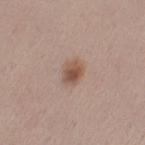Case summary:
* biopsy status — total-body-photography surveillance lesion; no biopsy
* tile lighting — white-light illumination
* size — about 2.5 mm
* subject — female, aged around 25
* site — the left thigh
* imaging modality — 15 mm crop, total-body photography